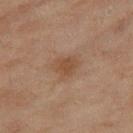Notes:
– follow-up · total-body-photography surveillance lesion; no biopsy
– body site · the right thigh
– image source · 15 mm crop, total-body photography
– diameter · about 3 mm
– lighting · cross-polarized illumination
– image-analysis metrics · a lesion area of about 6 mm², an eccentricity of roughly 0.55, and two-axis asymmetry of about 0.3; an average lesion color of about L≈43 a*≈17 b*≈29 (CIELAB) and a normalized lesion–skin contrast near 6.5; border irregularity of about 3 on a 0–10 scale, internal color variation of about 2 on a 0–10 scale, and peripheral color asymmetry of about 0.5
– patient · female, in their 60s